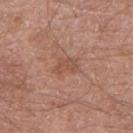<lesion>
  <biopsy_status>not biopsied; imaged during a skin examination</biopsy_status>
  <patient>
    <sex>male</sex>
    <age_approx>60</age_approx>
  </patient>
  <image>
    <source>total-body photography crop</source>
    <field_of_view_mm>15</field_of_view_mm>
  </image>
  <site>right upper arm</site>
</lesion>